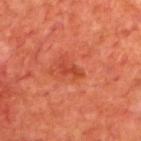{
  "biopsy_status": "not biopsied; imaged during a skin examination"
}